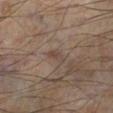Notes:
- biopsy status — catalogued during a skin exam; not biopsied
- tile lighting — cross-polarized illumination
- image — ~15 mm crop, total-body skin-cancer survey
- body site — the left lower leg
- patient — male, aged 53–57
- lesion size — ≈3 mm